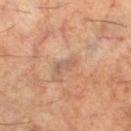Part of a total-body skin-imaging series; this lesion was reviewed on a skin check and was not flagged for biopsy. The lesion is located on the right lower leg. A male subject in their 60s. The tile uses cross-polarized illumination. A 15 mm close-up tile from a total-body photography series done for melanoma screening.